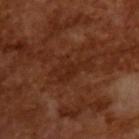Case summary:
• workup · catalogued during a skin exam; not biopsied
• subject · male, in their mid-60s
• imaging modality · ~15 mm crop, total-body skin-cancer survey